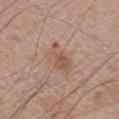workup: total-body-photography surveillance lesion; no biopsy | lighting: white-light illumination | image source: total-body-photography crop, ~15 mm field of view | location: the chest | TBP lesion metrics: an area of roughly 8 mm², an outline eccentricity of about 0.8 (0 = round, 1 = elongated), and two-axis asymmetry of about 0.35 | lesion size: ≈4 mm | patient: male, about 65 years old.